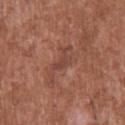{
  "biopsy_status": "not biopsied; imaged during a skin examination",
  "lesion_size": {
    "long_diameter_mm_approx": 2.5
  },
  "image": {
    "source": "total-body photography crop",
    "field_of_view_mm": 15
  },
  "site": "upper back",
  "patient": {
    "sex": "male",
    "age_approx": 45
  },
  "lighting": "white-light"
}